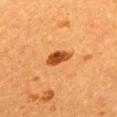The lesion was tiled from a total-body skin photograph and was not biopsied.
Cropped from a whole-body photographic skin survey; the tile spans about 15 mm.
The lesion is located on the mid back.
The lesion-visualizer software estimated a lesion color around L≈43 a*≈27 b*≈41 in CIELAB, roughly 16 lightness units darker than nearby skin, and a normalized border contrast of about 11.5. It also reported a peripheral color-asymmetry measure near 1.
A female patient, approximately 55 years of age.
The tile uses cross-polarized illumination.